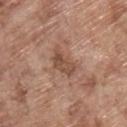Clinical impression: This lesion was catalogued during total-body skin photography and was not selected for biopsy. Context: A 15 mm close-up tile from a total-body photography series done for melanoma screening. From the upper back. This is a white-light tile. A male subject, roughly 70 years of age.